A female patient, aged 68 to 72 · cropped from a total-body skin-imaging series; the visible field is about 15 mm · from the lower back — 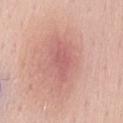Histopathology of the biopsied lesion showed a dysplastic (Clark) nevus.